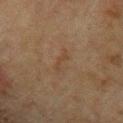workup — no biopsy performed (imaged during a skin exam).